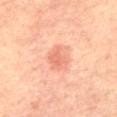| field | value |
|---|---|
| biopsy status | imaged on a skin check; not biopsied |
| site | the chest |
| automated lesion analysis | a shape eccentricity near 0.55 and a shape-asymmetry score of about 0.2 (0 = symmetric); a classifier nevus-likeness of about 85/100 |
| patient | female, approximately 65 years of age |
| size | ≈3 mm |
| imaging modality | ~15 mm crop, total-body skin-cancer survey |